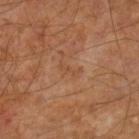Impression:
The lesion was photographed on a routine skin check and not biopsied; there is no pathology result.
Context:
From the right lower leg. Cropped from a whole-body photographic skin survey; the tile spans about 15 mm. A male subject, aged approximately 60.